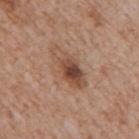| feature | finding |
|---|---|
| workup | imaged on a skin check; not biopsied |
| diameter | ~5.5 mm (longest diameter) |
| tile lighting | white-light illumination |
| image source | ~15 mm crop, total-body skin-cancer survey |
| body site | the mid back |
| subject | male, about 65 years old |